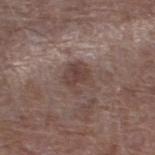No biopsy was performed on this lesion — it was imaged during a full skin examination and was not determined to be concerning. Located on the right lower leg. A male subject, roughly 70 years of age. A 15 mm close-up tile from a total-body photography series done for melanoma screening.